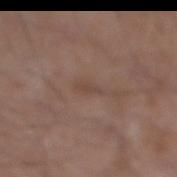Q: Was this lesion biopsied?
A: no biopsy performed (imaged during a skin exam)
Q: What are the patient's age and sex?
A: male, in their mid-50s
Q: What kind of image is this?
A: ~15 mm tile from a whole-body skin photo
Q: Lesion location?
A: the right lower leg
Q: What lighting was used for the tile?
A: white-light
Q: How large is the lesion?
A: ~3 mm (longest diameter)
Q: Automated lesion metrics?
A: an outline eccentricity of about 0.95 (0 = round, 1 = elongated); a mean CIELAB color near L≈44 a*≈17 b*≈23, roughly 6 lightness units darker than nearby skin, and a normalized border contrast of about 5; border irregularity of about 4.5 on a 0–10 scale and a within-lesion color-variation index near 0/10; a detector confidence of about 95 out of 100 that the crop contains a lesion